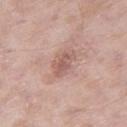{"biopsy_status": "not biopsied; imaged during a skin examination", "site": "leg", "automated_metrics": {"cielab_L": 58, "cielab_a": 20, "cielab_b": 25, "vs_skin_darker_L": 9.0, "vs_skin_contrast_norm": 6.0, "color_variation_0_10": 3.0, "peripheral_color_asymmetry": 1.0, "nevus_likeness_0_100": 0}, "image": {"source": "total-body photography crop", "field_of_view_mm": 15}, "lighting": "white-light", "patient": {"sex": "male", "age_approx": 55}, "lesion_size": {"long_diameter_mm_approx": 3.5}}